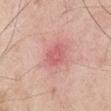* follow-up — no biopsy performed (imaged during a skin exam)
* patient — male, about 65 years old
* tile lighting — white-light
* site — the right upper arm
* image — ~15 mm crop, total-body skin-cancer survey